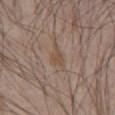The lesion was photographed on a routine skin check and not biopsied; there is no pathology result. Cropped from a total-body skin-imaging series; the visible field is about 15 mm. Longest diameter approximately 3 mm. The tile uses white-light illumination. From the chest. A male subject aged 63–67.